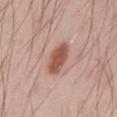Imaged during a routine full-body skin examination; the lesion was not biopsied and no histopathology is available. Measured at roughly 4 mm in maximum diameter. The lesion is on the mid back. A male patient, aged approximately 45. Cropped from a whole-body photographic skin survey; the tile spans about 15 mm. Automated tile analysis of the lesion measured a lesion color around L≈54 a*≈23 b*≈27 in CIELAB, roughly 13 lightness units darker than nearby skin, and a normalized lesion–skin contrast near 9.5.